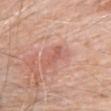Notes:
* notes — imaged on a skin check; not biopsied
* acquisition — total-body-photography crop, ~15 mm field of view
* illumination — white-light illumination
* patient — male, about 80 years old
* diameter — about 3 mm
* TBP lesion metrics — a border-irregularity index near 3/10 and peripheral color asymmetry of about 0
* anatomic site — the abdomen